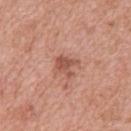Case summary:
- biopsy status · total-body-photography surveillance lesion; no biopsy
- site · the upper back
- image source · ~15 mm crop, total-body skin-cancer survey
- patient · female, aged approximately 40